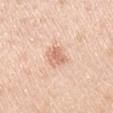workup = total-body-photography surveillance lesion; no biopsy
tile lighting = white-light illumination
subject = male, in their mid-40s
location = the arm
image source = ~15 mm tile from a whole-body skin photo
lesion diameter = about 3 mm